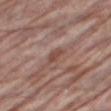follow-up = total-body-photography surveillance lesion; no biopsy
subject = male, aged around 70
image source = total-body-photography crop, ~15 mm field of view
body site = the right thigh